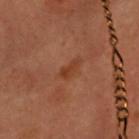Captured during whole-body skin photography for melanoma surveillance; the lesion was not biopsied. From the chest. A female subject, in their 50s. A roughly 15 mm field-of-view crop from a total-body skin photograph. Approximately 2.5 mm at its widest.